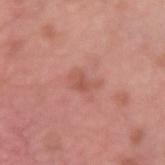follow-up=no biopsy performed (imaged during a skin exam)
location=the left forearm
subject=male, about 40 years old
imaging modality=total-body-photography crop, ~15 mm field of view
tile lighting=white-light illumination